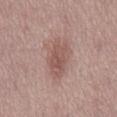The lesion was photographed on a routine skin check and not biopsied; there is no pathology result. The tile uses white-light illumination. A 15 mm close-up extracted from a 3D total-body photography capture. Located on the mid back. A male subject, aged 53 to 57.